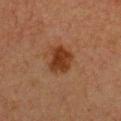Assessment: The lesion was photographed on a routine skin check and not biopsied; there is no pathology result. Background: The lesion's longest dimension is about 3.5 mm. This image is a 15 mm lesion crop taken from a total-body photograph. The tile uses cross-polarized illumination. The lesion is on the chest. A female patient in their 40s.